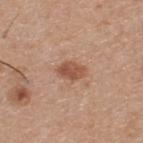A 15 mm close-up tile from a total-body photography series done for melanoma screening.
Imaged with white-light lighting.
On the upper back.
The total-body-photography lesion software estimated a footprint of about 5.5 mm², an outline eccentricity of about 0.75 (0 = round, 1 = elongated), and two-axis asymmetry of about 0.25. And it measured roughly 11 lightness units darker than nearby skin and a normalized lesion–skin contrast near 8. The software also gave a within-lesion color-variation index near 2.5/10.
A male subject in their 60s.